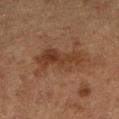  biopsy_status: not biopsied; imaged during a skin examination
  patient:
    sex: male
    age_approx: 75
  site: leg
  image:
    source: total-body photography crop
    field_of_view_mm: 15